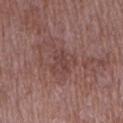Recorded during total-body skin imaging; not selected for excision or biopsy. Imaged with white-light lighting. This image is a 15 mm lesion crop taken from a total-body photograph. A female patient in their 70s. Measured at roughly 2.5 mm in maximum diameter. On the right upper arm.